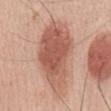follow-up: total-body-photography surveillance lesion; no biopsy
subject: male, aged 53 to 57
imaging modality: ~15 mm crop, total-body skin-cancer survey
location: the mid back